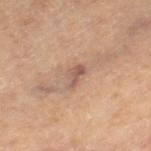anatomic site: the leg
lighting: cross-polarized illumination
image source: ~15 mm crop, total-body skin-cancer survey
subject: male, in their 60s
lesion diameter: ≈3 mm